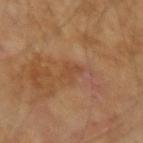Q: Was this lesion biopsied?
A: no biopsy performed (imaged during a skin exam)
Q: What kind of image is this?
A: ~15 mm crop, total-body skin-cancer survey
Q: Where on the body is the lesion?
A: the left upper arm
Q: Patient demographics?
A: aged 53–57
Q: What did automated image analysis measure?
A: a lesion area of about 3 mm² and two-axis asymmetry of about 0.35; an average lesion color of about L≈45 a*≈22 b*≈34 (CIELAB), roughly 6 lightness units darker than nearby skin, and a normalized border contrast of about 5; border irregularity of about 4 on a 0–10 scale and a color-variation rating of about 0.5/10; an automated nevus-likeness rating near 0 out of 100 and a lesion-detection confidence of about 100/100